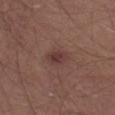notes: catalogued during a skin exam; not biopsied
anatomic site: the left thigh
diameter: about 3 mm
tile lighting: white-light illumination
subject: male, in their mid- to late 20s
image source: ~15 mm tile from a whole-body skin photo